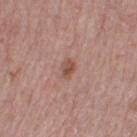Imaged during a routine full-body skin examination; the lesion was not biopsied and no histopathology is available.
On the right thigh.
A 15 mm close-up extracted from a 3D total-body photography capture.
Approximately 2.5 mm at its widest.
A female subject, approximately 40 years of age.
The lesion-visualizer software estimated an area of roughly 3 mm² and a shape eccentricity near 0.8.
Imaged with white-light lighting.